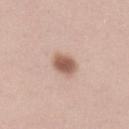biopsy status — imaged on a skin check; not biopsied | anatomic site — the arm | illumination — white-light illumination | patient — female, aged around 40 | image source — ~15 mm tile from a whole-body skin photo.